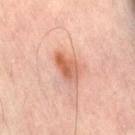Impression:
Captured during whole-body skin photography for melanoma surveillance; the lesion was not biopsied.
Background:
A 15 mm crop from a total-body photograph taken for skin-cancer surveillance. A male patient in their mid- to late 60s. About 4 mm across. Located on the right thigh. Automated image analysis of the tile measured an average lesion color of about L≈59 a*≈25 b*≈33 (CIELAB), roughly 11 lightness units darker than nearby skin, and a lesion-to-skin contrast of about 8 (normalized; higher = more distinct). It also reported a border-irregularity index near 2.5/10 and a within-lesion color-variation index near 6.5/10. Captured under cross-polarized illumination.